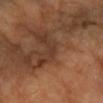{"site": "left arm", "image": {"source": "total-body photography crop", "field_of_view_mm": 15}, "patient": {"sex": "female", "age_approx": 60}}